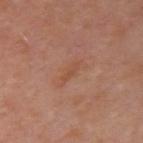The lesion was photographed on a routine skin check and not biopsied; there is no pathology result. The patient is a female aged approximately 50. The lesion-visualizer software estimated a footprint of about 2.5 mm², an eccentricity of roughly 0.95, and a symmetry-axis asymmetry near 0.35. The analysis additionally found border irregularity of about 4 on a 0–10 scale, a within-lesion color-variation index near 0/10, and peripheral color asymmetry of about 0. The analysis additionally found an automated nevus-likeness rating near 0 out of 100. Located on the left upper arm. Approximately 3 mm at its widest. Imaged with cross-polarized lighting. A close-up tile cropped from a whole-body skin photograph, about 15 mm across.